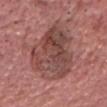workup: catalogued during a skin exam; not biopsied | site: the head or neck | image: ~15 mm crop, total-body skin-cancer survey | illumination: white-light illumination | patient: male, roughly 60 years of age.